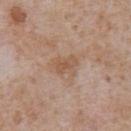notes=no biopsy performed (imaged during a skin exam)
site=the chest
image source=~15 mm tile from a whole-body skin photo
lighting=white-light illumination
lesion diameter=about 3.5 mm
subject=male, aged around 65